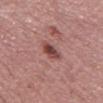This lesion was catalogued during total-body skin photography and was not selected for biopsy. From the left thigh. A roughly 15 mm field-of-view crop from a total-body skin photograph. Captured under white-light illumination. Approximately 3 mm at its widest. The subject is a female approximately 50 years of age.